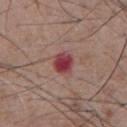workup=imaged on a skin check; not biopsied | image=~15 mm crop, total-body skin-cancer survey | diameter=≈3 mm | lighting=white-light illumination | site=the chest | patient=male, approximately 55 years of age.